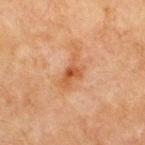Impression:
Imaged during a routine full-body skin examination; the lesion was not biopsied and no histopathology is available.
Acquisition and patient details:
Imaged with cross-polarized lighting. A 15 mm crop from a total-body photograph taken for skin-cancer surveillance. From the chest. Automated tile analysis of the lesion measured a shape eccentricity near 0.9. The software also gave a lesion color around L≈45 a*≈21 b*≈32 in CIELAB, a lesion–skin lightness drop of about 7, and a normalized lesion–skin contrast near 6.5. A male subject, about 65 years old. About 4.5 mm across.